The lesion's longest dimension is about 3.5 mm · the lesion is located on the mid back · imaged with cross-polarized lighting · a region of skin cropped from a whole-body photographic capture, roughly 15 mm wide · a male patient, about 70 years old:
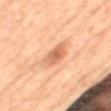Diagnosis:
On excision, pathology confirmed an invasive melanoma, Breslow thickness 0.5 mm and mitotic rate 0/mm².